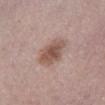The lesion was tiled from a total-body skin photograph and was not biopsied. A 15 mm close-up tile from a total-body photography series done for melanoma screening. A female patient about 40 years old. This is a white-light tile. Located on the right lower leg. The total-body-photography lesion software estimated a lesion area of about 10 mm², an outline eccentricity of about 0.55 (0 = round, 1 = elongated), and two-axis asymmetry of about 0.15. The analysis additionally found a lesion color around L≈53 a*≈19 b*≈24 in CIELAB, roughly 11 lightness units darker than nearby skin, and a normalized border contrast of about 8. The analysis additionally found border irregularity of about 1.5 on a 0–10 scale, a within-lesion color-variation index near 4/10, and radial color variation of about 1. The analysis additionally found an automated nevus-likeness rating near 70 out of 100 and lesion-presence confidence of about 100/100.